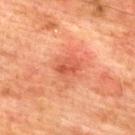workup: catalogued during a skin exam; not biopsied | illumination: cross-polarized illumination | lesion size: ~2.5 mm (longest diameter) | anatomic site: the upper back | image: 15 mm crop, total-body photography | subject: male, in their mid- to late 70s.